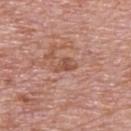{
  "biopsy_status": "not biopsied; imaged during a skin examination",
  "image": {
    "source": "total-body photography crop",
    "field_of_view_mm": 15
  },
  "site": "back",
  "lighting": "white-light",
  "patient": {
    "sex": "male",
    "age_approx": 70
  },
  "lesion_size": {
    "long_diameter_mm_approx": 3.0
  }
}